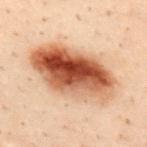• notes · imaged on a skin check; not biopsied
• imaging modality · ~15 mm crop, total-body skin-cancer survey
• location · the mid back
• TBP lesion metrics · a lesion area of about 39 mm², an eccentricity of roughly 0.85, and a symmetry-axis asymmetry near 0.1; about 17 CIELAB-L* units darker than the surrounding skin and a normalized lesion–skin contrast near 12.5; border irregularity of about 2 on a 0–10 scale, a color-variation rating of about 10/10, and a peripheral color-asymmetry measure near 5
• patient · male, aged around 30